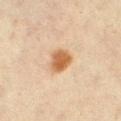Clinical summary:
A female subject, about 45 years old. From the left lower leg. A 15 mm close-up extracted from a 3D total-body photography capture. The tile uses cross-polarized illumination.